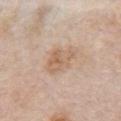Captured during whole-body skin photography for melanoma surveillance; the lesion was not biopsied.
A female patient in their mid-60s.
This is a white-light tile.
Automated image analysis of the tile measured a footprint of about 9.5 mm², a shape eccentricity near 0.75, and two-axis asymmetry of about 0.2. It also reported a lesion color around L≈62 a*≈16 b*≈31 in CIELAB, about 8 CIELAB-L* units darker than the surrounding skin, and a normalized border contrast of about 6. The software also gave a classifier nevus-likeness of about 0/100 and a detector confidence of about 100 out of 100 that the crop contains a lesion.
The lesion is on the chest.
A lesion tile, about 15 mm wide, cut from a 3D total-body photograph.